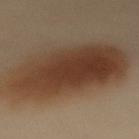Recorded during total-body skin imaging; not selected for excision or biopsy.
Located on the mid back.
A roughly 15 mm field-of-view crop from a total-body skin photograph.
A female subject, roughly 60 years of age.
The lesion's longest dimension is about 11.5 mm.
Imaged with cross-polarized lighting.
An algorithmic analysis of the crop reported a mean CIELAB color near L≈33 a*≈14 b*≈25, roughly 10 lightness units darker than nearby skin, and a normalized lesion–skin contrast near 10. It also reported a border-irregularity rating of about 2/10, a color-variation rating of about 5.5/10, and radial color variation of about 1.5.